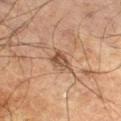Q: Was a biopsy performed?
A: no biopsy performed (imaged during a skin exam)
Q: Who is the patient?
A: male, about 70 years old
Q: What is the anatomic site?
A: the left thigh
Q: What is the imaging modality?
A: ~15 mm tile from a whole-body skin photo
Q: Lesion size?
A: ≈3 mm
Q: What lighting was used for the tile?
A: cross-polarized